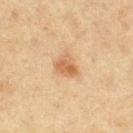Assessment: Part of a total-body skin-imaging series; this lesion was reviewed on a skin check and was not flagged for biopsy. Acquisition and patient details: An algorithmic analysis of the crop reported a lesion area of about 5 mm² and an outline eccentricity of about 0.55 (0 = round, 1 = elongated). And it measured a mean CIELAB color near L≈52 a*≈19 b*≈34 and about 9 CIELAB-L* units darker than the surrounding skin. The analysis additionally found border irregularity of about 2.5 on a 0–10 scale, a within-lesion color-variation index near 3/10, and a peripheral color-asymmetry measure near 1. The analysis additionally found a classifier nevus-likeness of about 95/100 and a lesion-detection confidence of about 100/100. This is a cross-polarized tile. The lesion is on the left thigh. A female patient approximately 50 years of age. This image is a 15 mm lesion crop taken from a total-body photograph.